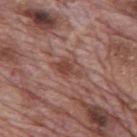A male patient, aged around 70.
Cropped from a total-body skin-imaging series; the visible field is about 15 mm.
Captured under white-light illumination.
Located on the back.